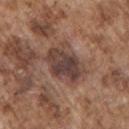notes: imaged on a skin check; not biopsied.